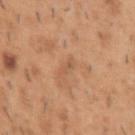{"biopsy_status": "not biopsied; imaged during a skin examination", "lesion_size": {"long_diameter_mm_approx": 2.5}, "image": {"source": "total-body photography crop", "field_of_view_mm": 15}, "lighting": "white-light", "site": "left upper arm", "patient": {"sex": "male", "age_approx": 40}}